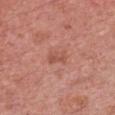Findings:
- notes: catalogued during a skin exam; not biopsied
- lighting: white-light illumination
- diameter: ~2.5 mm (longest diameter)
- acquisition: total-body-photography crop, ~15 mm field of view
- subject: female, aged 58–62
- anatomic site: the chest